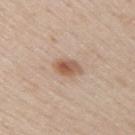The lesion was tiled from a total-body skin photograph and was not biopsied.
A 15 mm close-up tile from a total-body photography series done for melanoma screening.
A male subject, aged 48–52.
The lesion is located on the right upper arm.